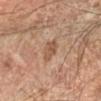Q: Is there a histopathology result?
A: total-body-photography surveillance lesion; no biopsy
Q: Patient demographics?
A: male, aged 58 to 62
Q: How was the tile lit?
A: cross-polarized
Q: What is the imaging modality?
A: ~15 mm crop, total-body skin-cancer survey
Q: Where on the body is the lesion?
A: the arm